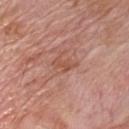workup=catalogued during a skin exam; not biopsied
diameter=about 3.5 mm
image source=15 mm crop, total-body photography
lighting=white-light illumination
subject=male, aged 58–62
anatomic site=the chest